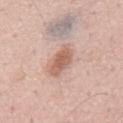| key | value |
|---|---|
| notes | catalogued during a skin exam; not biopsied |
| TBP lesion metrics | an eccentricity of roughly 0.9 and a shape-asymmetry score of about 0.3 (0 = symmetric); a lesion color around L≈61 a*≈21 b*≈28 in CIELAB, a lesion–skin lightness drop of about 12, and a normalized lesion–skin contrast near 7.5 |
| imaging modality | total-body-photography crop, ~15 mm field of view |
| tile lighting | white-light |
| patient | male, approximately 55 years of age |